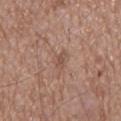The patient is a male in their mid-70s.
A roughly 15 mm field-of-view crop from a total-body skin photograph.
From the mid back.